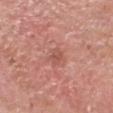<lesion>
  <biopsy_status>not biopsied; imaged during a skin examination</biopsy_status>
  <lesion_size>
    <long_diameter_mm_approx>2.5</long_diameter_mm_approx>
  </lesion_size>
  <image>
    <source>total-body photography crop</source>
    <field_of_view_mm>15</field_of_view_mm>
  </image>
  <patient>
    <sex>male</sex>
    <age_approx>60</age_approx>
  </patient>
  <automated_metrics>
    <border_irregularity_0_10>3.5</border_irregularity_0_10>
    <color_variation_0_10>1.0</color_variation_0_10>
    <nevus_likeness_0_100>0</nevus_likeness_0_100>
    <lesion_detection_confidence_0_100>100</lesion_detection_confidence_0_100>
  </automated_metrics>
  <lighting>white-light</lighting>
  <site>head or neck</site>
</lesion>